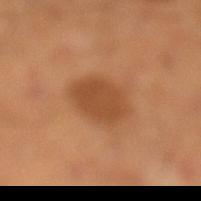No biopsy was performed on this lesion — it was imaged during a full skin examination and was not determined to be concerning.
The subject is a male in their 40s.
A 15 mm crop from a total-body photograph taken for skin-cancer surveillance.
The lesion is located on the leg.